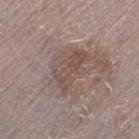{"biopsy_status": "not biopsied; imaged during a skin examination", "image": {"source": "total-body photography crop", "field_of_view_mm": 15}, "automated_metrics": {"peripheral_color_asymmetry": 1.0}, "site": "left thigh", "lighting": "white-light", "lesion_size": {"long_diameter_mm_approx": 5.5}, "patient": {"sex": "female", "age_approx": 65}}